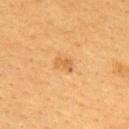workup — catalogued during a skin exam; not biopsied
tile lighting — cross-polarized
imaging modality — 15 mm crop, total-body photography
location — the right upper arm
subject — female, aged 38 to 42